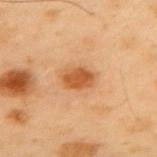{"biopsy_status": "not biopsied; imaged during a skin examination", "image": {"source": "total-body photography crop", "field_of_view_mm": 15}, "automated_metrics": {"border_irregularity_0_10": 1.5, "color_variation_0_10": 2.0, "nevus_likeness_0_100": 95, "lesion_detection_confidence_0_100": 100}, "lesion_size": {"long_diameter_mm_approx": 3.5}, "patient": {"sex": "male", "age_approx": 55}, "site": "back", "lighting": "cross-polarized"}